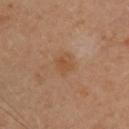Impression: The lesion was tiled from a total-body skin photograph and was not biopsied. Clinical summary: A 15 mm close-up extracted from a 3D total-body photography capture. Imaged with cross-polarized lighting. The lesion is located on the upper back. The subject is a female aged 33 to 37. Measured at roughly 2.5 mm in maximum diameter.